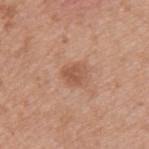No biopsy was performed on this lesion — it was imaged during a full skin examination and was not determined to be concerning. Cropped from a whole-body photographic skin survey; the tile spans about 15 mm. The subject is a male roughly 35 years of age. The lesion is located on the left upper arm. The lesion's longest dimension is about 2.5 mm. This is a white-light tile.